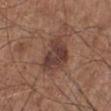Part of a total-body skin-imaging series; this lesion was reviewed on a skin check and was not flagged for biopsy. The total-body-photography lesion software estimated an area of roughly 13 mm², an outline eccentricity of about 0.7 (0 = round, 1 = elongated), and two-axis asymmetry of about 0.35. The software also gave a mean CIELAB color near L≈39 a*≈19 b*≈23 and roughly 10 lightness units darker than nearby skin. The software also gave a border-irregularity index near 3.5/10 and a color-variation rating of about 4/10. And it measured a nevus-likeness score of about 15/100 and lesion-presence confidence of about 100/100. Located on the leg. About 5 mm across. A male patient aged 58–62. The tile uses white-light illumination. A close-up tile cropped from a whole-body skin photograph, about 15 mm across.